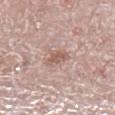Automated image analysis of the tile measured an area of roughly 4.5 mm², an eccentricity of roughly 0.7, and two-axis asymmetry of about 0.35. A lesion tile, about 15 mm wide, cut from a 3D total-body photograph. The lesion's longest dimension is about 3 mm. A male subject, about 70 years old. Located on the right leg.